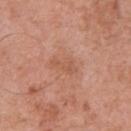Case summary:
- follow-up — total-body-photography surveillance lesion; no biopsy
- patient — male, aged 68–72
- automated metrics — about 6 CIELAB-L* units darker than the surrounding skin and a normalized border contrast of about 5; a border-irregularity index near 4/10; an automated nevus-likeness rating near 0 out of 100 and lesion-presence confidence of about 100/100
- imaging modality — 15 mm crop, total-body photography
- lesion size — ≈3.5 mm
- site — the chest